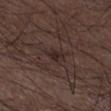Clinical summary:
Cropped from a whole-body photographic skin survey; the tile spans about 15 mm. From the left lower leg. The subject is a male in their 50s. This is a white-light tile.